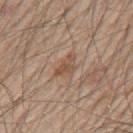No biopsy was performed on this lesion — it was imaged during a full skin examination and was not determined to be concerning.
This image is a 15 mm lesion crop taken from a total-body photograph.
Captured under white-light illumination.
The lesion is located on the mid back.
Automated tile analysis of the lesion measured a lesion color around L≈51 a*≈18 b*≈29 in CIELAB and a lesion-to-skin contrast of about 6.5 (normalized; higher = more distinct). The analysis additionally found a border-irregularity index near 5.5/10, a within-lesion color-variation index near 2/10, and a peripheral color-asymmetry measure near 0.5. And it measured an automated nevus-likeness rating near 0 out of 100 and a lesion-detection confidence of about 95/100.
The lesion's longest dimension is about 3 mm.
The patient is a male aged approximately 70.